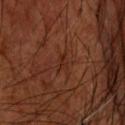The lesion was tiled from a total-body skin photograph and was not biopsied. A male subject, aged 58–62. Imaged with cross-polarized lighting. From the head or neck. A roughly 15 mm field-of-view crop from a total-body skin photograph. Longest diameter approximately 2.5 mm.